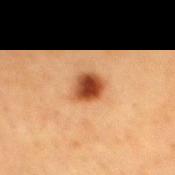On the mid back.
A 15 mm crop from a total-body photograph taken for skin-cancer surveillance.
A female subject, aged around 40.